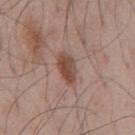Notes:
– diameter: ≈3.5 mm
– subject: male, roughly 55 years of age
– tile lighting: white-light illumination
– TBP lesion metrics: a border-irregularity index near 2/10 and radial color variation of about 1
– imaging modality: total-body-photography crop, ~15 mm field of view
– body site: the front of the torso